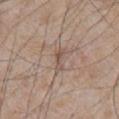Assessment: No biopsy was performed on this lesion — it was imaged during a full skin examination and was not determined to be concerning. Context: Automated tile analysis of the lesion measured about 8 CIELAB-L* units darker than the surrounding skin and a lesion-to-skin contrast of about 6 (normalized; higher = more distinct). The software also gave an automated nevus-likeness rating near 0 out of 100 and a detector confidence of about 90 out of 100 that the crop contains a lesion. The tile uses white-light illumination. A 15 mm close-up extracted from a 3D total-body photography capture. A male subject in their mid-60s. The lesion is on the chest.